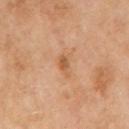Captured during whole-body skin photography for melanoma surveillance; the lesion was not biopsied.
Longest diameter approximately 2.5 mm.
From the arm.
A male patient aged around 70.
An algorithmic analysis of the crop reported an eccentricity of roughly 0.85 and two-axis asymmetry of about 0.35. And it measured a nevus-likeness score of about 0/100 and a detector confidence of about 100 out of 100 that the crop contains a lesion.
This image is a 15 mm lesion crop taken from a total-body photograph.